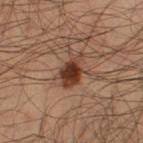Q: Was this lesion biopsied?
A: imaged on a skin check; not biopsied
Q: How was this image acquired?
A: total-body-photography crop, ~15 mm field of view
Q: What is the lesion's diameter?
A: about 4.5 mm
Q: Who is the patient?
A: male, aged 33–37
Q: Where on the body is the lesion?
A: the left thigh